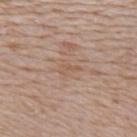Recorded during total-body skin imaging; not selected for excision or biopsy. The lesion is located on the upper back. Imaged with white-light lighting. Longest diameter approximately 2.5 mm. A lesion tile, about 15 mm wide, cut from a 3D total-body photograph. A male subject, roughly 65 years of age. Automated tile analysis of the lesion measured a footprint of about 2 mm² and two-axis asymmetry of about 0.6. The software also gave an automated nevus-likeness rating near 0 out of 100 and lesion-presence confidence of about 55/100.